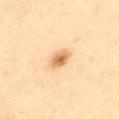– follow-up — imaged on a skin check; not biopsied
– imaging modality — 15 mm crop, total-body photography
– patient — female, in their 60s
– illumination — cross-polarized
– body site — the abdomen
– size — ≈2.5 mm
– image-analysis metrics — an average lesion color of about L≈72 a*≈23 b*≈44 (CIELAB), about 14 CIELAB-L* units darker than the surrounding skin, and a normalized border contrast of about 8.5; a classifier nevus-likeness of about 100/100 and lesion-presence confidence of about 100/100